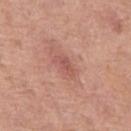<record>
  <biopsy_status>not biopsied; imaged during a skin examination</biopsy_status>
  <lighting>white-light</lighting>
  <image>
    <source>total-body photography crop</source>
    <field_of_view_mm>15</field_of_view_mm>
  </image>
  <site>left thigh</site>
  <lesion_size>
    <long_diameter_mm_approx>3.0</long_diameter_mm_approx>
  </lesion_size>
  <patient>
    <sex>female</sex>
    <age_approx>65</age_approx>
  </patient>
</record>